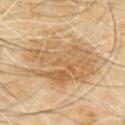follow-up=no biopsy performed (imaged during a skin exam); illumination=cross-polarized; lesion diameter=about 8.5 mm; anatomic site=the mid back; patient=male, about 60 years old; image=15 mm crop, total-body photography.